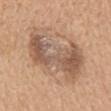A female patient, in their mid-50s.
On the mid back.
The lesion's longest dimension is about 8.5 mm.
A 15 mm close-up extracted from a 3D total-body photography capture.